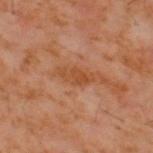Imaged during a routine full-body skin examination; the lesion was not biopsied and no histopathology is available.
This is a cross-polarized tile.
The lesion is on the back.
This image is a 15 mm lesion crop taken from a total-body photograph.
The subject is a male aged 58 to 62.
The lesion's longest dimension is about 3.5 mm.